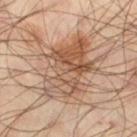workup: total-body-photography surveillance lesion; no biopsy
location: the left thigh
image: ~15 mm tile from a whole-body skin photo
patient: male, aged around 45